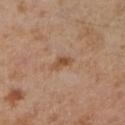A female subject aged 38–42.
The lesion is on the arm.
A roughly 15 mm field-of-view crop from a total-body skin photograph.
The tile uses cross-polarized illumination.